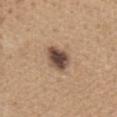Impression: Recorded during total-body skin imaging; not selected for excision or biopsy. Acquisition and patient details: The lesion is located on the back. Approximately 3.5 mm at its widest. This is a white-light tile. A roughly 15 mm field-of-view crop from a total-body skin photograph. The total-body-photography lesion software estimated an area of roughly 7.5 mm², an outline eccentricity of about 0.6 (0 = round, 1 = elongated), and a shape-asymmetry score of about 0.2 (0 = symmetric). And it measured a classifier nevus-likeness of about 60/100 and a detector confidence of about 100 out of 100 that the crop contains a lesion. The patient is a female aged around 60.